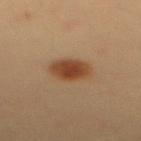The lesion was photographed on a routine skin check and not biopsied; there is no pathology result.
A female subject, in their 30s.
The lesion is located on the upper back.
A close-up tile cropped from a whole-body skin photograph, about 15 mm across.